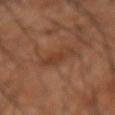| field | value |
|---|---|
| notes | no biopsy performed (imaged during a skin exam) |
| lesion size | ≈3.5 mm |
| anatomic site | the right forearm |
| tile lighting | cross-polarized |
| subject | male, about 45 years old |
| image | 15 mm crop, total-body photography |